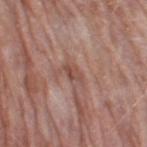No biopsy was performed on this lesion — it was imaged during a full skin examination and was not determined to be concerning. Located on the right thigh. This image is a 15 mm lesion crop taken from a total-body photograph. Imaged with white-light lighting. About 3 mm across. A female subject, approximately 70 years of age. Automated image analysis of the tile measured a footprint of about 3.5 mm², an outline eccentricity of about 0.85 (0 = round, 1 = elongated), and a symmetry-axis asymmetry near 0.25. The software also gave a mean CIELAB color near L≈49 a*≈22 b*≈25, about 8 CIELAB-L* units darker than the surrounding skin, and a normalized lesion–skin contrast near 6.5. The analysis additionally found a nevus-likeness score of about 0/100 and a detector confidence of about 85 out of 100 that the crop contains a lesion.